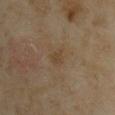A female patient about 35 years old.
The lesion-visualizer software estimated internal color variation of about 1 on a 0–10 scale and radial color variation of about 0.5. The software also gave a classifier nevus-likeness of about 0/100 and a lesion-detection confidence of about 100/100.
Longest diameter approximately 2.5 mm.
A 15 mm close-up tile from a total-body photography series done for melanoma screening.
On the upper back.